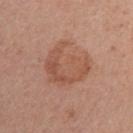Image and clinical context:
Approximately 5.5 mm at its widest. A female patient aged around 55. This is a white-light tile. A 15 mm crop from a total-body photograph taken for skin-cancer surveillance. Automated tile analysis of the lesion measured a mean CIELAB color near L≈53 a*≈23 b*≈30 and roughly 8 lightness units darker than nearby skin. The analysis additionally found an automated nevus-likeness rating near 15 out of 100 and lesion-presence confidence of about 100/100. Located on the right upper arm.